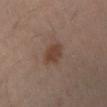A lesion tile, about 15 mm wide, cut from a 3D total-body photograph. Imaged with cross-polarized lighting. Automated tile analysis of the lesion measured an average lesion color of about L≈38 a*≈16 b*≈24 (CIELAB) and a lesion-to-skin contrast of about 7.5 (normalized; higher = more distinct). It also reported border irregularity of about 2 on a 0–10 scale, a color-variation rating of about 2/10, and a peripheral color-asymmetry measure near 0.5. The software also gave an automated nevus-likeness rating near 45 out of 100 and a lesion-detection confidence of about 100/100. A male patient aged around 65. From the left lower leg.